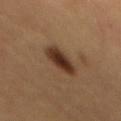workup: total-body-photography surveillance lesion; no biopsy | lesion size: ≈4.5 mm | patient: male, aged around 60 | body site: the mid back | image: 15 mm crop, total-body photography | TBP lesion metrics: a lesion color around L≈29 a*≈16 b*≈25 in CIELAB, roughly 12 lightness units darker than nearby skin, and a normalized border contrast of about 11.5; a color-variation rating of about 3.5/10 and radial color variation of about 1; a nevus-likeness score of about 100/100 and a detector confidence of about 100 out of 100 that the crop contains a lesion.